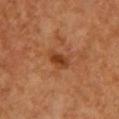Case summary:
* biopsy status — imaged on a skin check; not biopsied
* imaging modality — ~15 mm tile from a whole-body skin photo
* automated metrics — a lesion color around L≈43 a*≈26 b*≈38 in CIELAB, roughly 10 lightness units darker than nearby skin, and a lesion-to-skin contrast of about 7.5 (normalized; higher = more distinct); a classifier nevus-likeness of about 45/100 and a detector confidence of about 100 out of 100 that the crop contains a lesion
* lesion size — ~3 mm (longest diameter)
* location — the chest
* subject — female, aged around 55